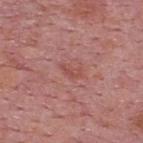Assessment: No biopsy was performed on this lesion — it was imaged during a full skin examination and was not determined to be concerning. Clinical summary: The lesion is on the upper back. About 3 mm across. This is a white-light tile. A male patient, aged 73 to 77. A 15 mm close-up extracted from a 3D total-body photography capture.